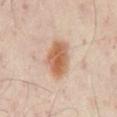lighting: cross-polarized illumination
size: ~4.5 mm (longest diameter)
site: the chest
subject: male, approximately 50 years of age
automated lesion analysis: an average lesion color of about L≈60 a*≈21 b*≈33 (CIELAB), about 13 CIELAB-L* units darker than the surrounding skin, and a lesion-to-skin contrast of about 9 (normalized; higher = more distinct)
imaging modality: ~15 mm crop, total-body skin-cancer survey